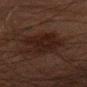Case summary:
– follow-up — imaged on a skin check; not biopsied
– subject — male, in their 80s
– image — ~15 mm crop, total-body skin-cancer survey
– location — the right thigh
– lighting — cross-polarized
– lesion diameter — about 5.5 mm